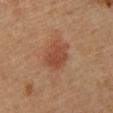A roughly 15 mm field-of-view crop from a total-body skin photograph.
The lesion-visualizer software estimated a footprint of about 8 mm² and a shape eccentricity near 0.5. And it measured a color-variation rating of about 1.5/10 and peripheral color asymmetry of about 0.5.
This is a cross-polarized tile.
A male subject, about 60 years old.
Located on the mid back.
Measured at roughly 3.5 mm in maximum diameter.